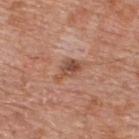Impression: No biopsy was performed on this lesion — it was imaged during a full skin examination and was not determined to be concerning. Background: Imaged with white-light lighting. The lesion-visualizer software estimated an area of roughly 5 mm², an eccentricity of roughly 0.85, and a shape-asymmetry score of about 0.45 (0 = symmetric). It also reported about 10 CIELAB-L* units darker than the surrounding skin. The software also gave border irregularity of about 4.5 on a 0–10 scale and peripheral color asymmetry of about 1.5. It also reported a nevus-likeness score of about 5/100 and lesion-presence confidence of about 100/100. A region of skin cropped from a whole-body photographic capture, roughly 15 mm wide. The lesion is on the upper back. A male patient, about 80 years old. Measured at roughly 4 mm in maximum diameter.